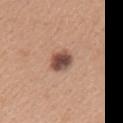biopsy status = catalogued during a skin exam; not biopsied | subject = female, in their 30s | site = the left upper arm | automated lesion analysis = a lesion color around L≈49 a*≈21 b*≈26 in CIELAB and a normalized lesion–skin contrast near 11.5; a border-irregularity rating of about 2/10; a detector confidence of about 100 out of 100 that the crop contains a lesion | lighting = white-light | image = 15 mm crop, total-body photography.